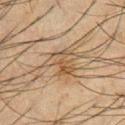biopsy status — no biopsy performed (imaged during a skin exam)
subject — male, about 35 years old
body site — the chest
image source — ~15 mm tile from a whole-body skin photo
automated lesion analysis — an area of roughly 8.5 mm², a shape eccentricity near 0.55, and two-axis asymmetry of about 0.5; a lesion-to-skin contrast of about 6 (normalized; higher = more distinct)